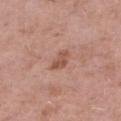Impression:
Captured during whole-body skin photography for melanoma surveillance; the lesion was not biopsied.
Context:
A female patient aged around 60. A 15 mm crop from a total-body photograph taken for skin-cancer surveillance. On the right thigh.